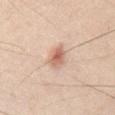Assessment:
Captured during whole-body skin photography for melanoma surveillance; the lesion was not biopsied.
Context:
A region of skin cropped from a whole-body photographic capture, roughly 15 mm wide. On the chest. The subject is a male aged 43 to 47.